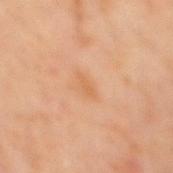Case summary:
* workup: catalogued during a skin exam; not biopsied
* subject: male, roughly 60 years of age
* image: ~15 mm crop, total-body skin-cancer survey
* site: the mid back